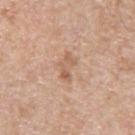<lesion>
<biopsy_status>not biopsied; imaged during a skin examination</biopsy_status>
<site>upper back</site>
<image>
  <source>total-body photography crop</source>
  <field_of_view_mm>15</field_of_view_mm>
</image>
<lighting>white-light</lighting>
<lesion_size>
  <long_diameter_mm_approx>3.0</long_diameter_mm_approx>
</lesion_size>
<patient>
  <sex>male</sex>
  <age_approx>55</age_approx>
</patient>
</lesion>